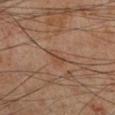Clinical impression: This lesion was catalogued during total-body skin photography and was not selected for biopsy. Context: About 2.5 mm across. Automated tile analysis of the lesion measured roughly 7 lightness units darker than nearby skin. The software also gave border irregularity of about 4 on a 0–10 scale, a color-variation rating of about 0.5/10, and radial color variation of about 0. The analysis additionally found lesion-presence confidence of about 80/100. The subject is a male aged 68–72. A lesion tile, about 15 mm wide, cut from a 3D total-body photograph. On the left lower leg.